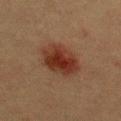{
  "biopsy_status": "not biopsied; imaged during a skin examination",
  "site": "chest",
  "image": {
    "source": "total-body photography crop",
    "field_of_view_mm": 15
  },
  "patient": {
    "sex": "male",
    "age_approx": 40
  }
}